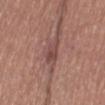{"biopsy_status": "not biopsied; imaged during a skin examination", "image": {"source": "total-body photography crop", "field_of_view_mm": 15}, "patient": {"sex": "female", "age_approx": 55}, "lesion_size": {"long_diameter_mm_approx": 4.0}, "site": "right thigh", "lighting": "white-light"}